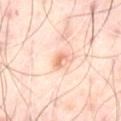patient:
  sex: male
  age_approx: 50
image:
  source: total-body photography crop
  field_of_view_mm: 15
site: abdomen